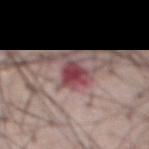A lesion tile, about 15 mm wide, cut from a 3D total-body photograph.
Located on the front of the torso.
The lesion-visualizer software estimated a lesion area of about 6 mm² and a shape-asymmetry score of about 0.3 (0 = symmetric).
The lesion's longest dimension is about 3.5 mm.
The subject is a male aged 58 to 62.
Imaged with white-light lighting.